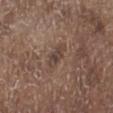size: about 3 mm
lighting: white-light
location: the left lower leg
imaging modality: total-body-photography crop, ~15 mm field of view
patient: male, roughly 70 years of age